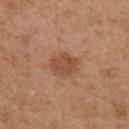Clinical impression: The lesion was photographed on a routine skin check and not biopsied; there is no pathology result. Background: A female subject in their 30s. Captured under white-light illumination. Automated tile analysis of the lesion measured a lesion area of about 8 mm² and a shape-asymmetry score of about 0.2 (0 = symmetric). And it measured a lesion color around L≈49 a*≈22 b*≈32 in CIELAB and roughly 9 lightness units darker than nearby skin. The analysis additionally found a nevus-likeness score of about 55/100 and a lesion-detection confidence of about 100/100. A region of skin cropped from a whole-body photographic capture, roughly 15 mm wide. About 3.5 mm across. The lesion is on the arm.